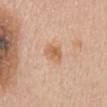Part of a total-body skin-imaging series; this lesion was reviewed on a skin check and was not flagged for biopsy. The lesion is on the chest. The tile uses white-light illumination. Approximately 2.5 mm at its widest. A female subject, in their mid-60s. Cropped from a whole-body photographic skin survey; the tile spans about 15 mm.